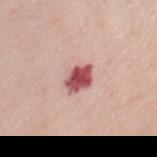Findings:
– workup: imaged on a skin check; not biopsied
– subject: female, aged approximately 55
– anatomic site: the front of the torso
– acquisition: ~15 mm crop, total-body skin-cancer survey